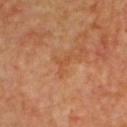image source: total-body-photography crop, ~15 mm field of view
size: ≈2.5 mm
site: the back
subject: male, roughly 60 years of age
TBP lesion metrics: a lesion area of about 2.5 mm² and an outline eccentricity of about 0.8 (0 = round, 1 = elongated)
tile lighting: cross-polarized